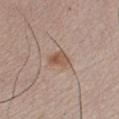No biopsy was performed on this lesion — it was imaged during a full skin examination and was not determined to be concerning.
From the chest.
The tile uses white-light illumination.
Approximately 2.5 mm at its widest.
A male patient about 45 years old.
This image is a 15 mm lesion crop taken from a total-body photograph.